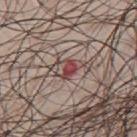Imaged during a routine full-body skin examination; the lesion was not biopsied and no histopathology is available. A male subject, in their mid-40s. A roughly 15 mm field-of-view crop from a total-body skin photograph. The lesion is located on the chest.